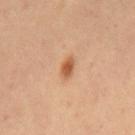Q: Is there a histopathology result?
A: no biopsy performed (imaged during a skin exam)
Q: Patient demographics?
A: male, roughly 60 years of age
Q: What lighting was used for the tile?
A: cross-polarized
Q: What is the lesion's diameter?
A: about 2.5 mm
Q: What is the imaging modality?
A: ~15 mm tile from a whole-body skin photo
Q: What did automated image analysis measure?
A: a lesion area of about 3.5 mm², an outline eccentricity of about 0.85 (0 = round, 1 = elongated), and a symmetry-axis asymmetry near 0.3
Q: What is the anatomic site?
A: the mid back